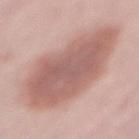{"patient": {"sex": "male", "age_approx": 70}, "site": "lower back", "lesion_size": {"long_diameter_mm_approx": 10.5}, "lighting": "white-light", "image": {"source": "total-body photography crop", "field_of_view_mm": 15}}